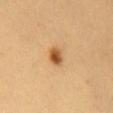Impression: Imaged during a routine full-body skin examination; the lesion was not biopsied and no histopathology is available. Image and clinical context: The lesion is on the chest. The tile uses cross-polarized illumination. The lesion-visualizer software estimated a mean CIELAB color near L≈56 a*≈23 b*≈43, a lesion–skin lightness drop of about 14, and a normalized border contrast of about 9.5. And it measured a border-irregularity rating of about 1.5/10, a within-lesion color-variation index near 6/10, and a peripheral color-asymmetry measure near 2. The software also gave an automated nevus-likeness rating near 100 out of 100 and a lesion-detection confidence of about 100/100. Approximately 2.5 mm at its widest. Cropped from a whole-body photographic skin survey; the tile spans about 15 mm. A female patient, aged approximately 35.